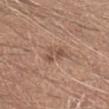Q: Was a biopsy performed?
A: total-body-photography surveillance lesion; no biopsy
Q: What lighting was used for the tile?
A: white-light illumination
Q: How large is the lesion?
A: ≈3 mm
Q: What is the anatomic site?
A: the left forearm
Q: Who is the patient?
A: male, roughly 25 years of age
Q: How was this image acquired?
A: ~15 mm tile from a whole-body skin photo